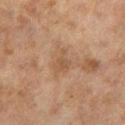| key | value |
|---|---|
| biopsy status | no biopsy performed (imaged during a skin exam) |
| diameter | ≈2.5 mm |
| anatomic site | the left lower leg |
| lighting | cross-polarized illumination |
| automated metrics | a border-irregularity rating of about 5.5/10, a color-variation rating of about 1.5/10, and radial color variation of about 0.5; a nevus-likeness score of about 0/100 and lesion-presence confidence of about 100/100 |
| image | 15 mm crop, total-body photography |
| subject | female, aged 58–62 |